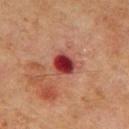Impression: Imaged during a routine full-body skin examination; the lesion was not biopsied and no histopathology is available. Context: A 15 mm crop from a total-body photograph taken for skin-cancer surveillance. The lesion's longest dimension is about 3.5 mm. The subject is a male aged 68–72. Located on the back.